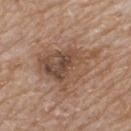workup: no biopsy performed (imaged during a skin exam)
diameter: about 7.5 mm
automated metrics: a lesion area of about 24 mm², an outline eccentricity of about 0.7 (0 = round, 1 = elongated), and two-axis asymmetry of about 0.35; a lesion color around L≈49 a*≈17 b*≈28 in CIELAB and a normalized border contrast of about 7.5; a border-irregularity index near 5/10 and internal color variation of about 7.5 on a 0–10 scale; an automated nevus-likeness rating near 20 out of 100 and a detector confidence of about 100 out of 100 that the crop contains a lesion
illumination: white-light
anatomic site: the upper back
image: ~15 mm crop, total-body skin-cancer survey
patient: male, approximately 80 years of age